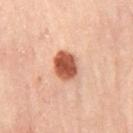{
  "biopsy_status": "not biopsied; imaged during a skin examination",
  "site": "right thigh",
  "patient": {
    "sex": "female",
    "age_approx": 60
  },
  "lighting": "cross-polarized",
  "image": {
    "source": "total-body photography crop",
    "field_of_view_mm": 15
  }
}